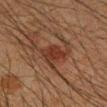Notes:
* follow-up · imaged on a skin check; not biopsied
* body site · the right forearm
* image · 15 mm crop, total-body photography
* subject · male, aged around 35